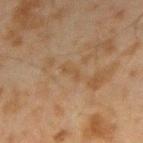This lesion was catalogued during total-body skin photography and was not selected for biopsy.
This image is a 15 mm lesion crop taken from a total-body photograph.
From the right forearm.
A male subject about 45 years old.
The lesion's longest dimension is about 2.5 mm.